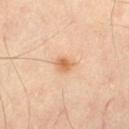• biopsy status · imaged on a skin check; not biopsied
• subject · male, aged approximately 55
• size · about 2.5 mm
• image source · ~15 mm tile from a whole-body skin photo
• lighting · cross-polarized
• body site · the left thigh